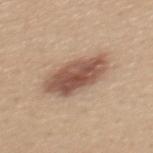Q: Is there a histopathology result?
A: imaged on a skin check; not biopsied
Q: What is the anatomic site?
A: the upper back
Q: Patient demographics?
A: female, about 30 years old
Q: How was this image acquired?
A: 15 mm crop, total-body photography
Q: How was the tile lit?
A: white-light illumination
Q: Lesion size?
A: about 6.5 mm
Q: Automated lesion metrics?
A: a footprint of about 18 mm², a shape eccentricity near 0.85, and two-axis asymmetry of about 0.2; about 15 CIELAB-L* units darker than the surrounding skin and a normalized lesion–skin contrast near 10; a border-irregularity index near 2.5/10, internal color variation of about 5.5 on a 0–10 scale, and a peripheral color-asymmetry measure near 2; an automated nevus-likeness rating near 95 out of 100